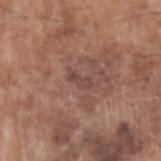Clinical summary: The lesion's longest dimension is about 2.5 mm. The subject is a male in their mid-70s. On the left upper arm. Imaged with white-light lighting. A 15 mm close-up extracted from a 3D total-body photography capture.